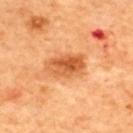Captured during whole-body skin photography for melanoma surveillance; the lesion was not biopsied.
A 15 mm close-up tile from a total-body photography series done for melanoma screening.
From the back.
A male patient, aged 43–47.